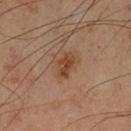Part of a total-body skin-imaging series; this lesion was reviewed on a skin check and was not flagged for biopsy. A male subject, in their mid-50s. A 15 mm close-up extracted from a 3D total-body photography capture. The tile uses cross-polarized illumination. Located on the left lower leg. About 3 mm across.